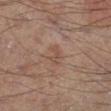notes=catalogued during a skin exam; not biopsied | subject=male, approximately 55 years of age | size=~3 mm (longest diameter) | tile lighting=cross-polarized illumination | acquisition=~15 mm crop, total-body skin-cancer survey | body site=the left lower leg.